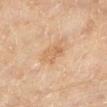Measured at roughly 3 mm in maximum diameter. From the left lower leg. Automated image analysis of the tile measured a lesion–skin lightness drop of about 6 and a normalized lesion–skin contrast near 5. The software also gave a nevus-likeness score of about 0/100. A lesion tile, about 15 mm wide, cut from a 3D total-body photograph. The tile uses cross-polarized illumination. The subject is a female aged around 55.